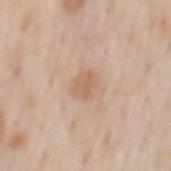| field | value |
|---|---|
| notes | total-body-photography surveillance lesion; no biopsy |
| lesion size | ~2.5 mm (longest diameter) |
| image | 15 mm crop, total-body photography |
| body site | the mid back |
| lighting | white-light illumination |
| patient | male, aged 58 to 62 |